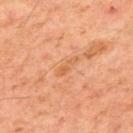workup: total-body-photography surveillance lesion; no biopsy | subject: male, approximately 60 years of age | lighting: cross-polarized illumination | image source: ~15 mm crop, total-body skin-cancer survey | size: ≈3 mm | site: the upper back | automated metrics: a lesion area of about 2.5 mm², a shape eccentricity near 0.95, and a shape-asymmetry score of about 0.35 (0 = symmetric); an average lesion color of about L≈58 a*≈25 b*≈40 (CIELAB) and about 6 CIELAB-L* units darker than the surrounding skin; border irregularity of about 3.5 on a 0–10 scale, internal color variation of about 0 on a 0–10 scale, and a peripheral color-asymmetry measure near 0; a detector confidence of about 100 out of 100 that the crop contains a lesion.